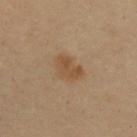Clinical impression: Part of a total-body skin-imaging series; this lesion was reviewed on a skin check and was not flagged for biopsy.